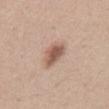– workup — total-body-photography surveillance lesion; no biopsy
– lesion diameter — ≈3.5 mm
– imaging modality — ~15 mm crop, total-body skin-cancer survey
– site — the abdomen
– patient — female, aged 43–47
– illumination — white-light